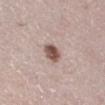An algorithmic analysis of the crop reported a footprint of about 5 mm², an outline eccentricity of about 0.65 (0 = round, 1 = elongated), and a symmetry-axis asymmetry near 0.15. It also reported a lesion color around L≈52 a*≈18 b*≈22 in CIELAB, roughly 16 lightness units darker than nearby skin, and a normalized border contrast of about 10.5. It also reported a border-irregularity rating of about 1/10, internal color variation of about 4.5 on a 0–10 scale, and peripheral color asymmetry of about 1.5. A roughly 15 mm field-of-view crop from a total-body skin photograph. On the right lower leg. The tile uses white-light illumination. Longest diameter approximately 2.5 mm. The patient is a female aged 53 to 57.